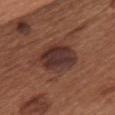About 5.5 mm across. From the chest. A female patient about 65 years old. Captured under white-light illumination. Automated image analysis of the tile measured an automated nevus-likeness rating near 70 out of 100 and a detector confidence of about 100 out of 100 that the crop contains a lesion. A 15 mm close-up tile from a total-body photography series done for melanoma screening.